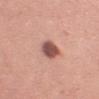Findings:
* workup — catalogued during a skin exam; not biopsied
* TBP lesion metrics — a mean CIELAB color near L≈52 a*≈24 b*≈24, a lesion–skin lightness drop of about 17, and a normalized lesion–skin contrast near 11; a border-irregularity index near 2/10, internal color variation of about 4 on a 0–10 scale, and peripheral color asymmetry of about 1.5; lesion-presence confidence of about 100/100
* subject — female, about 40 years old
* size — ≈3.5 mm
* image source — ~15 mm crop, total-body skin-cancer survey
* site — the right upper arm